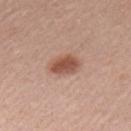Recorded during total-body skin imaging; not selected for excision or biopsy. From the left upper arm. The subject is a male approximately 55 years of age. Captured under white-light illumination. A region of skin cropped from a whole-body photographic capture, roughly 15 mm wide. The lesion's longest dimension is about 3.5 mm.